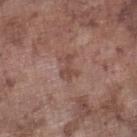The lesion was photographed on a routine skin check and not biopsied; there is no pathology result.
This image is a 15 mm lesion crop taken from a total-body photograph.
The tile uses white-light illumination.
On the left lower leg.
Measured at roughly 3 mm in maximum diameter.
A male patient in their mid- to late 70s.
An algorithmic analysis of the crop reported an area of roughly 3 mm² and a symmetry-axis asymmetry near 0.65. The software also gave a lesion color around L≈46 a*≈20 b*≈23 in CIELAB, roughly 8 lightness units darker than nearby skin, and a normalized lesion–skin contrast near 6. And it measured a border-irregularity index near 7.5/10 and internal color variation of about 0 on a 0–10 scale. The analysis additionally found a classifier nevus-likeness of about 0/100 and a lesion-detection confidence of about 100/100.